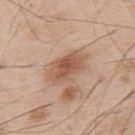<case>
<biopsy_status>not biopsied; imaged during a skin examination</biopsy_status>
<patient>
  <sex>male</sex>
  <age_approx>55</age_approx>
</patient>
<lesion_size>
  <long_diameter_mm_approx>5.0</long_diameter_mm_approx>
</lesion_size>
<lighting>white-light</lighting>
<image>
  <source>total-body photography crop</source>
  <field_of_view_mm>15</field_of_view_mm>
</image>
<site>upper back</site>
<automated_metrics>
  <cielab_L>55</cielab_L>
  <cielab_a>21</cielab_a>
  <cielab_b>30</cielab_b>
  <vs_skin_darker_L>11.0</vs_skin_darker_L>
  <vs_skin_contrast_norm>7.5</vs_skin_contrast_norm>
  <border_irregularity_0_10>2.5</border_irregularity_0_10>
  <color_variation_0_10>4.0</color_variation_0_10>
  <peripheral_color_asymmetry>1.0</peripheral_color_asymmetry>
  <nevus_likeness_0_100>75</nevus_likeness_0_100>
  <lesion_detection_confidence_0_100>100</lesion_detection_confidence_0_100>
</automated_metrics>
</case>